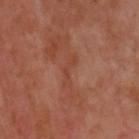workup: no biopsy performed (imaged during a skin exam)
acquisition: total-body-photography crop, ~15 mm field of view
illumination: cross-polarized illumination
patient: male, in their mid-50s
size: ≈3.5 mm
TBP lesion metrics: a lesion area of about 3 mm² and an eccentricity of roughly 0.95; a border-irregularity rating of about 7.5/10, a within-lesion color-variation index near 0/10, and peripheral color asymmetry of about 0
location: the upper back